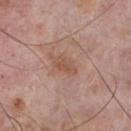<record>
  <biopsy_status>not biopsied; imaged during a skin examination</biopsy_status>
  <patient>
    <sex>male</sex>
    <age_approx>75</age_approx>
  </patient>
  <image>
    <source>total-body photography crop</source>
    <field_of_view_mm>15</field_of_view_mm>
  </image>
  <lesion_size>
    <long_diameter_mm_approx>3.0</long_diameter_mm_approx>
  </lesion_size>
  <automated_metrics>
    <vs_skin_darker_L>7.0</vs_skin_darker_L>
    <vs_skin_contrast_norm>5.5</vs_skin_contrast_norm>
    <border_irregularity_0_10>2.0</border_irregularity_0_10>
    <color_variation_0_10>1.5</color_variation_0_10>
    <peripheral_color_asymmetry>0.5</peripheral_color_asymmetry>
  </automated_metrics>
  <site>front of the torso</site>
  <lighting>white-light</lighting>
</record>